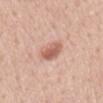<record>
  <biopsy_status>not biopsied; imaged during a skin examination</biopsy_status>
  <patient>
    <sex>male</sex>
    <age_approx>55</age_approx>
  </patient>
  <image>
    <source>total-body photography crop</source>
    <field_of_view_mm>15</field_of_view_mm>
  </image>
  <lesion_size>
    <long_diameter_mm_approx>3.0</long_diameter_mm_approx>
  </lesion_size>
  <site>back</site>
  <lighting>white-light</lighting>
</record>